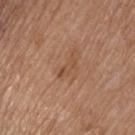Assessment: Captured during whole-body skin photography for melanoma surveillance; the lesion was not biopsied.